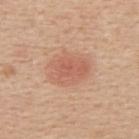Background: Cropped from a total-body skin-imaging series; the visible field is about 15 mm. From the upper back. Captured under white-light illumination. A female patient, approximately 50 years of age. Longest diameter approximately 5 mm.